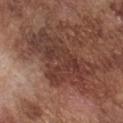This lesion was catalogued during total-body skin photography and was not selected for biopsy.
The patient is a male aged 73 to 77.
The lesion is on the chest.
A 15 mm crop from a total-body photograph taken for skin-cancer surveillance.